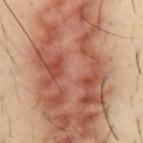Notes:
- follow-up — no biopsy performed (imaged during a skin exam)
- diameter — ~21 mm (longest diameter)
- subject — male, roughly 30 years of age
- image — total-body-photography crop, ~15 mm field of view
- illumination — cross-polarized illumination
- anatomic site — the abdomen